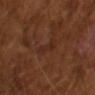| feature | finding |
|---|---|
| biopsy status | imaged on a skin check; not biopsied |
| image | ~15 mm tile from a whole-body skin photo |
| illumination | cross-polarized illumination |
| subject | male, in their mid- to late 60s |
| lesion size | ~4 mm (longest diameter) |